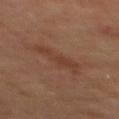notes: imaged on a skin check; not biopsied | patient: male, in their 70s | automated metrics: a lesion area of about 8.5 mm² and two-axis asymmetry of about 0.35 | acquisition: ~15 mm tile from a whole-body skin photo | anatomic site: the mid back.